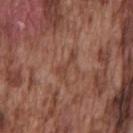biopsy_status: not biopsied; imaged during a skin examination
patient:
  sex: male
  age_approx: 75
site: front of the torso
image:
  source: total-body photography crop
  field_of_view_mm: 15
lesion_size:
  long_diameter_mm_approx: 3.5
lighting: white-light